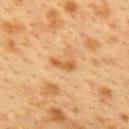  biopsy_status: not biopsied; imaged during a skin examination
  lesion_size:
    long_diameter_mm_approx: 3.0
  lighting: cross-polarized
  image:
    source: total-body photography crop
    field_of_view_mm: 15
  patient:
    sex: female
    age_approx: 40
  automated_metrics:
    area_mm2_approx: 3.0
    eccentricity: 0.95
    shape_asymmetry: 0.3
    border_irregularity_0_10: 4.0
    color_variation_0_10: 0.5
    nevus_likeness_0_100: 0
  site: upper back